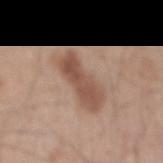workup = catalogued during a skin exam; not biopsied
lighting = white-light
anatomic site = the mid back
imaging modality = total-body-photography crop, ~15 mm field of view
patient = male, roughly 45 years of age
lesion size = about 6 mm